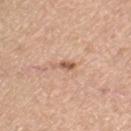The lesion was photographed on a routine skin check and not biopsied; there is no pathology result.
Automated image analysis of the tile measured an area of roughly 2.5 mm², an outline eccentricity of about 0.85 (0 = round, 1 = elongated), and a shape-asymmetry score of about 0.4 (0 = symmetric). The software also gave an average lesion color of about L≈59 a*≈21 b*≈31 (CIELAB), about 12 CIELAB-L* units darker than the surrounding skin, and a normalized border contrast of about 7.5. The analysis additionally found a border-irregularity rating of about 4/10, a within-lesion color-variation index near 1.5/10, and a peripheral color-asymmetry measure near 0. The software also gave a lesion-detection confidence of about 100/100.
Cropped from a total-body skin-imaging series; the visible field is about 15 mm.
The lesion's longest dimension is about 2.5 mm.
A female subject, about 70 years old.
The lesion is on the left thigh.